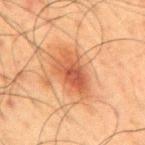No biopsy was performed on this lesion — it was imaged during a full skin examination and was not determined to be concerning.